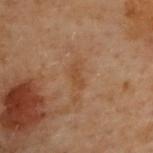Impression: No biopsy was performed on this lesion — it was imaged during a full skin examination and was not determined to be concerning. Background: A 15 mm close-up extracted from a 3D total-body photography capture. A female subject, aged 58 to 62. The lesion's longest dimension is about 2.5 mm. The lesion is on the upper back.